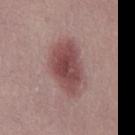| key | value |
|---|---|
| notes | imaged on a skin check; not biopsied |
| image source | ~15 mm tile from a whole-body skin photo |
| patient | male, roughly 30 years of age |
| illumination | white-light |
| lesion diameter | about 6.5 mm |